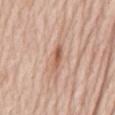The lesion was tiled from a total-body skin photograph and was not biopsied.
A female patient about 65 years old.
Measured at roughly 3.5 mm in maximum diameter.
The lesion is located on the mid back.
A lesion tile, about 15 mm wide, cut from a 3D total-body photograph.